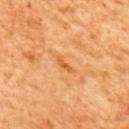Assessment:
Captured during whole-body skin photography for melanoma surveillance; the lesion was not biopsied.
Context:
A female subject aged 63–67. Located on the upper back. The total-body-photography lesion software estimated a footprint of about 2 mm² and an outline eccentricity of about 0.95 (0 = round, 1 = elongated). The software also gave border irregularity of about 4.5 on a 0–10 scale, a color-variation rating of about 0/10, and peripheral color asymmetry of about 0. It also reported a detector confidence of about 100 out of 100 that the crop contains a lesion. This is a cross-polarized tile. Measured at roughly 2.5 mm in maximum diameter. This image is a 15 mm lesion crop taken from a total-body photograph.